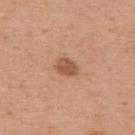  biopsy_status: not biopsied; imaged during a skin examination
  lighting: white-light
  site: upper back
  lesion_size:
    long_diameter_mm_approx: 2.5
  image:
    source: total-body photography crop
    field_of_view_mm: 15
  patient:
    sex: female
    age_approx: 40